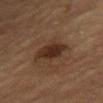Impression:
Part of a total-body skin-imaging series; this lesion was reviewed on a skin check and was not flagged for biopsy.
Acquisition and patient details:
From the chest. A female subject aged approximately 70. A 15 mm crop from a total-body photograph taken for skin-cancer surveillance. Captured under cross-polarized illumination. Longest diameter approximately 4 mm. The total-body-photography lesion software estimated a mean CIELAB color near L≈24 a*≈15 b*≈22. It also reported a border-irregularity rating of about 2.5/10.